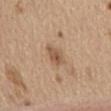follow-up = no biopsy performed (imaged during a skin exam) | patient = male, aged 68 to 72 | location = the abdomen | size = about 3 mm | acquisition = 15 mm crop, total-body photography.